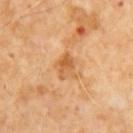Recorded during total-body skin imaging; not selected for excision or biopsy.
A 15 mm crop from a total-body photograph taken for skin-cancer surveillance.
The subject is a male about 65 years old.
The tile uses cross-polarized illumination.
Approximately 3.5 mm at its widest.